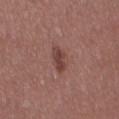Clinical impression: Imaged during a routine full-body skin examination; the lesion was not biopsied and no histopathology is available. Clinical summary: Automated image analysis of the tile measured a nevus-likeness score of about 60/100 and a lesion-detection confidence of about 100/100. The tile uses white-light illumination. A 15 mm close-up extracted from a 3D total-body photography capture. Located on the left thigh. The patient is a female in their 40s.